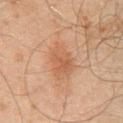follow-up: total-body-photography surveillance lesion; no biopsy.